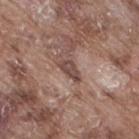follow-up = catalogued during a skin exam; not biopsied
lesion diameter = about 3.5 mm
image = 15 mm crop, total-body photography
patient = male, about 75 years old
anatomic site = the right thigh
tile lighting = white-light illumination